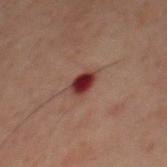| feature | finding |
|---|---|
| workup | imaged on a skin check; not biopsied |
| patient | male, about 60 years old |
| imaging modality | ~15 mm crop, total-body skin-cancer survey |
| body site | the front of the torso |